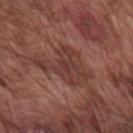{"biopsy_status": "not biopsied; imaged during a skin examination", "lighting": "white-light", "site": "right upper arm", "patient": {"sex": "male", "age_approx": 75}, "image": {"source": "total-body photography crop", "field_of_view_mm": 15}, "lesion_size": {"long_diameter_mm_approx": 6.5}}